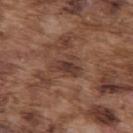Notes:
- follow-up — imaged on a skin check; not biopsied
- TBP lesion metrics — a lesion color around L≈38 a*≈19 b*≈25 in CIELAB, a lesion–skin lightness drop of about 8, and a normalized lesion–skin contrast near 7.5; border irregularity of about 4 on a 0–10 scale and a color-variation rating of about 3.5/10; a nevus-likeness score of about 0/100 and a detector confidence of about 75 out of 100 that the crop contains a lesion
- site — the upper back
- image source — ~15 mm tile from a whole-body skin photo
- patient — male, roughly 75 years of age